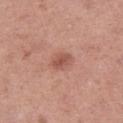{
  "biopsy_status": "not biopsied; imaged during a skin examination",
  "lighting": "white-light",
  "site": "right thigh",
  "lesion_size": {
    "long_diameter_mm_approx": 2.5
  },
  "image": {
    "source": "total-body photography crop",
    "field_of_view_mm": 15
  },
  "automated_metrics": {
    "area_mm2_approx": 4.5,
    "eccentricity": 0.75,
    "shape_asymmetry": 0.25,
    "cielab_L": 53,
    "cielab_a": 25,
    "cielab_b": 28,
    "vs_skin_darker_L": 10.0,
    "vs_skin_contrast_norm": 6.5,
    "nevus_likeness_0_100": 65,
    "lesion_detection_confidence_0_100": 100
  },
  "patient": {
    "sex": "female",
    "age_approx": 40
  }
}